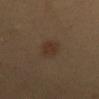This lesion was catalogued during total-body skin photography and was not selected for biopsy. Approximately 3 mm at its widest. From the abdomen. Captured under cross-polarized illumination. A close-up tile cropped from a whole-body skin photograph, about 15 mm across. An algorithmic analysis of the crop reported a lesion area of about 5.5 mm² and a symmetry-axis asymmetry near 0.3. It also reported a lesion color around L≈32 a*≈14 b*≈26 in CIELAB, roughly 6 lightness units darker than nearby skin, and a normalized lesion–skin contrast near 6.5. It also reported a classifier nevus-likeness of about 80/100. A female subject roughly 55 years of age.